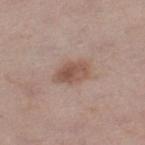<case>
<biopsy_status>not biopsied; imaged during a skin examination</biopsy_status>
<image>
  <source>total-body photography crop</source>
  <field_of_view_mm>15</field_of_view_mm>
</image>
<site>leg</site>
<lesion_size>
  <long_diameter_mm_approx>4.0</long_diameter_mm_approx>
</lesion_size>
<patient>
  <sex>female</sex>
  <age_approx>40</age_approx>
</patient>
<lighting>white-light</lighting>
</case>